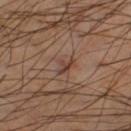Clinical impression: Captured during whole-body skin photography for melanoma surveillance; the lesion was not biopsied. Background: A male patient roughly 50 years of age. Measured at roughly 2.5 mm in maximum diameter. Automated tile analysis of the lesion measured a border-irregularity index near 5.5/10 and a peripheral color-asymmetry measure near 0. The tile uses cross-polarized illumination. A close-up tile cropped from a whole-body skin photograph, about 15 mm across. The lesion is on the right lower leg.